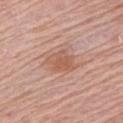biopsy status: catalogued during a skin exam; not biopsied
image: 15 mm crop, total-body photography
patient: male, aged 78–82
lesion diameter: ~4 mm (longest diameter)
automated lesion analysis: a footprint of about 8 mm² and a shape-asymmetry score of about 0.2 (0 = symmetric); a mean CIELAB color near L≈57 a*≈22 b*≈30, about 8 CIELAB-L* units darker than the surrounding skin, and a normalized border contrast of about 6
tile lighting: white-light
site: the left upper arm